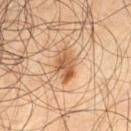Q: Where on the body is the lesion?
A: the left thigh
Q: How large is the lesion?
A: ~4 mm (longest diameter)
Q: What kind of image is this?
A: 15 mm crop, total-body photography
Q: What are the patient's age and sex?
A: male, aged around 55
Q: What lighting was used for the tile?
A: cross-polarized